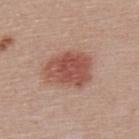Assessment:
This lesion was catalogued during total-body skin photography and was not selected for biopsy.
Background:
Longest diameter approximately 5.5 mm. Imaged with white-light lighting. The lesion is on the upper back. A lesion tile, about 15 mm wide, cut from a 3D total-body photograph. A male subject about 40 years old.